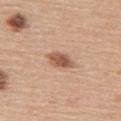Assessment: Captured during whole-body skin photography for melanoma surveillance; the lesion was not biopsied. Clinical summary: A female subject, approximately 65 years of age. Cropped from a total-body skin-imaging series; the visible field is about 15 mm. Located on the back. The recorded lesion diameter is about 3.5 mm. Imaged with white-light lighting.